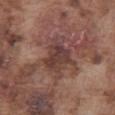lighting: white-light
image:
  source: total-body photography crop
  field_of_view_mm: 15
site: abdomen
lesion_size:
  long_diameter_mm_approx: 4.0
patient:
  sex: male
  age_approx: 75